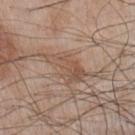Assessment:
Recorded during total-body skin imaging; not selected for excision or biopsy.
Clinical summary:
The lesion is located on the chest. This image is a 15 mm lesion crop taken from a total-body photograph. The lesion-visualizer software estimated a border-irregularity rating of about 5.5/10, a within-lesion color-variation index near 4/10, and peripheral color asymmetry of about 1.5. The subject is a male roughly 65 years of age.